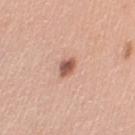Notes:
- notes · total-body-photography surveillance lesion; no biopsy
- lesion diameter · ≈2.5 mm
- illumination · white-light
- image · total-body-photography crop, ~15 mm field of view
- patient · male, aged approximately 60
- anatomic site · the left upper arm